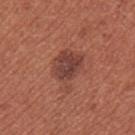biopsy_status: not biopsied; imaged during a skin examination
site: right upper arm
lesion_size:
  long_diameter_mm_approx: 4.5
image:
  source: total-body photography crop
  field_of_view_mm: 15
patient:
  sex: female
  age_approx: 35
lighting: white-light